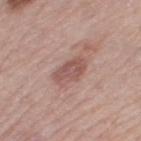Findings:
- follow-up — no biopsy performed (imaged during a skin exam)
- site — the leg
- acquisition — ~15 mm tile from a whole-body skin photo
- subject — female, aged 63 to 67